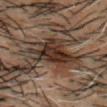| field | value |
|---|---|
| follow-up | total-body-photography surveillance lesion; no biopsy |
| body site | the head or neck |
| patient | male, about 50 years old |
| image source | 15 mm crop, total-body photography |